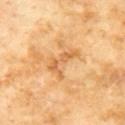Q: Was this lesion biopsied?
A: catalogued during a skin exam; not biopsied
Q: What lighting was used for the tile?
A: cross-polarized illumination
Q: What is the anatomic site?
A: the chest
Q: How was this image acquired?
A: ~15 mm crop, total-body skin-cancer survey
Q: What are the patient's age and sex?
A: male, aged 58 to 62
Q: What is the lesion's diameter?
A: ~4 mm (longest diameter)
Q: Automated lesion metrics?
A: a mean CIELAB color near L≈62 a*≈23 b*≈44, a lesion–skin lightness drop of about 9, and a lesion-to-skin contrast of about 6 (normalized; higher = more distinct); a border-irregularity index near 8/10, a color-variation rating of about 0.5/10, and a peripheral color-asymmetry measure near 0; a lesion-detection confidence of about 100/100